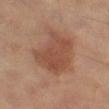Part of a total-body skin-imaging series; this lesion was reviewed on a skin check and was not flagged for biopsy.
This image is a 15 mm lesion crop taken from a total-body photograph.
A male subject, roughly 65 years of age.
The lesion is located on the left thigh.